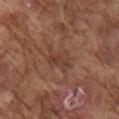Findings:
* follow-up: total-body-photography surveillance lesion; no biopsy
* acquisition: total-body-photography crop, ~15 mm field of view
* tile lighting: white-light illumination
* anatomic site: the right upper arm
* subject: male, roughly 75 years of age
* lesion size: ≈3.5 mm